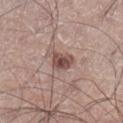This lesion was catalogued during total-body skin photography and was not selected for biopsy. From the right lower leg. A male subject aged approximately 50. A region of skin cropped from a whole-body photographic capture, roughly 15 mm wide. The recorded lesion diameter is about 3 mm. The total-body-photography lesion software estimated border irregularity of about 3.5 on a 0–10 scale, internal color variation of about 4 on a 0–10 scale, and a peripheral color-asymmetry measure near 1.5. And it measured a lesion-detection confidence of about 100/100. The tile uses white-light illumination.